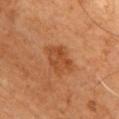Impression:
Part of a total-body skin-imaging series; this lesion was reviewed on a skin check and was not flagged for biopsy.
Context:
The subject is a male about 60 years old. The total-body-photography lesion software estimated a footprint of about 9 mm², an outline eccentricity of about 0.7 (0 = round, 1 = elongated), and a symmetry-axis asymmetry near 0.2. The software also gave a detector confidence of about 100 out of 100 that the crop contains a lesion. On the chest. This is a cross-polarized tile. Cropped from a whole-body photographic skin survey; the tile spans about 15 mm.